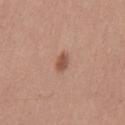<tbp_lesion>
  <biopsy_status>not biopsied; imaged during a skin examination</biopsy_status>
  <image>
    <source>total-body photography crop</source>
    <field_of_view_mm>15</field_of_view_mm>
  </image>
  <patient>
    <sex>male</sex>
    <age_approx>35</age_approx>
  </patient>
  <lesion_size>
    <long_diameter_mm_approx>2.5</long_diameter_mm_approx>
  </lesion_size>
  <site>chest</site>
  <automated_metrics>
    <area_mm2_approx>3.5</area_mm2_approx>
    <eccentricity>0.8</eccentricity>
    <shape_asymmetry>0.25</shape_asymmetry>
    <border_irregularity_0_10>2.5</border_irregularity_0_10>
    <color_variation_0_10>2.0</color_variation_0_10>
    <peripheral_color_asymmetry>0.5</peripheral_color_asymmetry>
  </automated_metrics>
  <lighting>white-light</lighting>
</tbp_lesion>